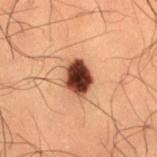The lesion was photographed on a routine skin check and not biopsied; there is no pathology result.
This image is a 15 mm lesion crop taken from a total-body photograph.
The tile uses cross-polarized illumination.
From the right thigh.
Measured at roughly 4 mm in maximum diameter.
A male patient aged around 50.